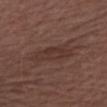Recorded during total-body skin imaging; not selected for excision or biopsy.
A 15 mm close-up tile from a total-body photography series done for melanoma screening.
The tile uses white-light illumination.
Approximately 5.5 mm at its widest.
The patient is a male aged approximately 70.
On the right upper arm.